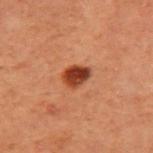workup — catalogued during a skin exam; not biopsied | image source — total-body-photography crop, ~15 mm field of view | lighting — cross-polarized | patient — male, aged approximately 60 | location — the left upper arm | TBP lesion metrics — a shape eccentricity near 0.5 and two-axis asymmetry of about 0.2; an average lesion color of about L≈32 a*≈24 b*≈29 (CIELAB), a lesion–skin lightness drop of about 13, and a lesion-to-skin contrast of about 12 (normalized; higher = more distinct); a within-lesion color-variation index near 4/10 and a peripheral color-asymmetry measure near 1.5; a classifier nevus-likeness of about 100/100 and a detector confidence of about 100 out of 100 that the crop contains a lesion | lesion size — about 3 mm.